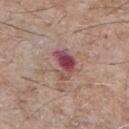Q: Was a biopsy performed?
A: total-body-photography surveillance lesion; no biopsy
Q: What kind of image is this?
A: ~15 mm tile from a whole-body skin photo
Q: Patient demographics?
A: male, aged 63–67
Q: Where on the body is the lesion?
A: the chest